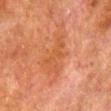Assessment: Captured during whole-body skin photography for melanoma surveillance; the lesion was not biopsied. Image and clinical context: Measured at roughly 6.5 mm in maximum diameter. A 15 mm crop from a total-body photograph taken for skin-cancer surveillance. The lesion is on the right lower leg. A male subject aged around 80. Imaged with cross-polarized lighting.